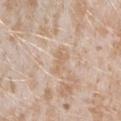The lesion was photographed on a routine skin check and not biopsied; there is no pathology result.
The tile uses white-light illumination.
The recorded lesion diameter is about 3 mm.
A female subject approximately 25 years of age.
A 15 mm close-up extracted from a 3D total-body photography capture.
Located on the right forearm.